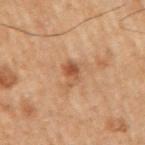Clinical impression:
Part of a total-body skin-imaging series; this lesion was reviewed on a skin check and was not flagged for biopsy.
Context:
On the right upper arm. The patient is a male aged approximately 70. A roughly 15 mm field-of-view crop from a total-body skin photograph.